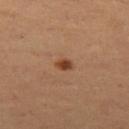The lesion was photographed on a routine skin check and not biopsied; there is no pathology result. A female patient, aged 53 to 57. A roughly 15 mm field-of-view crop from a total-body skin photograph. From the left thigh. The lesion-visualizer software estimated an average lesion color of about L≈43 a*≈23 b*≈34 (CIELAB), a lesion–skin lightness drop of about 12, and a normalized lesion–skin contrast near 9.5. The analysis additionally found a classifier nevus-likeness of about 95/100.